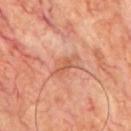<record>
<biopsy_status>not biopsied; imaged during a skin examination</biopsy_status>
<site>chest</site>
<automated_metrics>
  <vs_skin_darker_L>8.0</vs_skin_darker_L>
  <vs_skin_contrast_norm>6.0</vs_skin_contrast_norm>
  <nevus_likeness_0_100>0</nevus_likeness_0_100>
  <lesion_detection_confidence_0_100>100</lesion_detection_confidence_0_100>
</automated_metrics>
<lesion_size>
  <long_diameter_mm_approx>3.5</long_diameter_mm_approx>
</lesion_size>
<image>
  <source>total-body photography crop</source>
  <field_of_view_mm>15</field_of_view_mm>
</image>
<patient>
  <sex>male</sex>
  <age_approx>65</age_approx>
</patient>
</record>